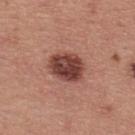Background:
The lesion-visualizer software estimated a border-irregularity rating of about 2/10 and peripheral color asymmetry of about 2. The software also gave an automated nevus-likeness rating near 55 out of 100 and a lesion-detection confidence of about 100/100. A male subject roughly 40 years of age. This is a white-light tile. A lesion tile, about 15 mm wide, cut from a 3D total-body photograph. Located on the upper back. The recorded lesion diameter is about 4.5 mm.